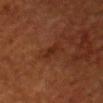The subject is a male about 55 years old. The lesion is located on the head or neck. An algorithmic analysis of the crop reported a shape eccentricity near 0.9 and two-axis asymmetry of about 0.3. The analysis additionally found a lesion-to-skin contrast of about 6 (normalized; higher = more distinct). A 15 mm close-up extracted from a 3D total-body photography capture.